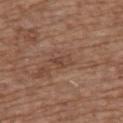{
  "site": "upper back",
  "image": {
    "source": "total-body photography crop",
    "field_of_view_mm": 15
  },
  "patient": {
    "sex": "female",
    "age_approx": 75
  }
}